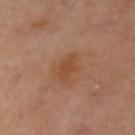Q: Is there a histopathology result?
A: imaged on a skin check; not biopsied
Q: How was this image acquired?
A: ~15 mm crop, total-body skin-cancer survey
Q: What is the lesion's diameter?
A: ~3 mm (longest diameter)
Q: How was the tile lit?
A: cross-polarized
Q: Lesion location?
A: the left upper arm